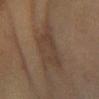This lesion was catalogued during total-body skin photography and was not selected for biopsy. The total-body-photography lesion software estimated a footprint of about 14 mm², a shape eccentricity near 0.7, and a shape-asymmetry score of about 0.4 (0 = symmetric). And it measured an average lesion color of about L≈35 a*≈13 b*≈23 (CIELAB), roughly 6 lightness units darker than nearby skin, and a normalized lesion–skin contrast near 6. And it measured a border-irregularity index near 4.5/10 and peripheral color asymmetry of about 1. It also reported a classifier nevus-likeness of about 0/100 and a detector confidence of about 95 out of 100 that the crop contains a lesion. Approximately 5.5 mm at its widest. The lesion is located on the left forearm. The patient is a female aged approximately 60. Imaged with cross-polarized lighting. A region of skin cropped from a whole-body photographic capture, roughly 15 mm wide.